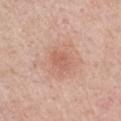| feature | finding |
|---|---|
| notes | imaged on a skin check; not biopsied |
| image | 15 mm crop, total-body photography |
| site | the left upper arm |
| subject | male, aged 53 to 57 |